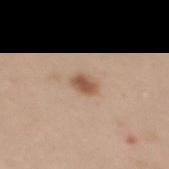Clinical impression: Captured during whole-body skin photography for melanoma surveillance; the lesion was not biopsied. Clinical summary: The recorded lesion diameter is about 2.5 mm. A female patient, about 25 years old. A roughly 15 mm field-of-view crop from a total-body skin photograph. Imaged with white-light lighting. An algorithmic analysis of the crop reported a footprint of about 4 mm² and a shape-asymmetry score of about 0.2 (0 = symmetric). It also reported a lesion color around L≈55 a*≈19 b*≈31 in CIELAB. The analysis additionally found a border-irregularity index near 1.5/10 and a within-lesion color-variation index near 2.5/10. The software also gave a classifier nevus-likeness of about 95/100 and lesion-presence confidence of about 100/100. On the upper back.